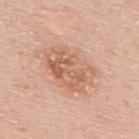Impression: No biopsy was performed on this lesion — it was imaged during a full skin examination and was not determined to be concerning. Acquisition and patient details: A male patient aged 48–52. The lesion is on the upper back. This image is a 15 mm lesion crop taken from a total-body photograph. The tile uses white-light illumination. The lesion's longest dimension is about 6 mm.